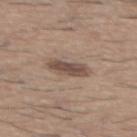Image and clinical context:
Located on the mid back. This image is a 15 mm lesion crop taken from a total-body photograph. The tile uses white-light illumination. The patient is a male aged 58 to 62. Longest diameter approximately 4 mm.